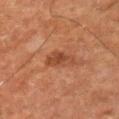Assessment: The lesion was tiled from a total-body skin photograph and was not biopsied. Background: The total-body-photography lesion software estimated a lesion color around L≈36 a*≈22 b*≈29 in CIELAB, roughly 8 lightness units darker than nearby skin, and a normalized lesion–skin contrast near 7. And it measured a border-irregularity rating of about 4/10, a within-lesion color-variation index near 2/10, and radial color variation of about 0.5. A 15 mm close-up tile from a total-body photography series done for melanoma screening. The tile uses cross-polarized illumination. On the left upper arm. A male subject, about 75 years old.